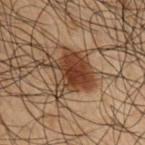workup: imaged on a skin check; not biopsied | anatomic site: the right upper arm | illumination: cross-polarized | patient: male, aged 48 to 52 | image-analysis metrics: a footprint of about 17 mm² and a symmetry-axis asymmetry near 0.25; a mean CIELAB color near L≈30 a*≈15 b*≈24 and a lesion-to-skin contrast of about 10.5 (normalized; higher = more distinct); an automated nevus-likeness rating near 95 out of 100 | size: ≈5 mm | image: 15 mm crop, total-body photography.